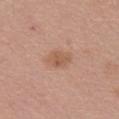This is a white-light tile. A 15 mm close-up extracted from a 3D total-body photography capture. The lesion is located on the chest. A female patient, aged 63 to 67. About 3 mm across.